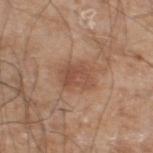Case summary:
- biopsy status: total-body-photography surveillance lesion; no biopsy
- acquisition: ~15 mm crop, total-body skin-cancer survey
- diameter: ~3.5 mm (longest diameter)
- site: the left lower leg
- subject: male, in their 60s